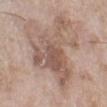Captured during whole-body skin photography for melanoma surveillance; the lesion was not biopsied.
A close-up tile cropped from a whole-body skin photograph, about 15 mm across.
On the left lower leg.
A female patient, aged 73–77.